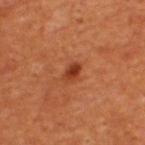{"biopsy_status": "not biopsied; imaged during a skin examination", "image": {"source": "total-body photography crop", "field_of_view_mm": 15}, "site": "back", "patient": {"sex": "male", "age_approx": 55}}